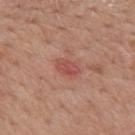Clinical impression: Captured during whole-body skin photography for melanoma surveillance; the lesion was not biopsied. Acquisition and patient details: A male patient aged around 55. The lesion is on the mid back. Captured under white-light illumination. Cropped from a whole-body photographic skin survey; the tile spans about 15 mm. The lesion's longest dimension is about 3 mm. Automated image analysis of the tile measured a footprint of about 4.5 mm², a shape eccentricity near 0.8, and two-axis asymmetry of about 0.25. The software also gave a lesion color around L≈51 a*≈28 b*≈26 in CIELAB, roughly 8 lightness units darker than nearby skin, and a normalized border contrast of about 6.